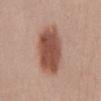Approximately 7 mm at its widest. A female patient aged 23–27. The lesion is on the abdomen. Automated tile analysis of the lesion measured a lesion area of about 21 mm², a shape eccentricity near 0.85, and two-axis asymmetry of about 0.1. It also reported a border-irregularity rating of about 2/10 and a within-lesion color-variation index near 3.5/10. A region of skin cropped from a whole-body photographic capture, roughly 15 mm wide. The tile uses white-light illumination.